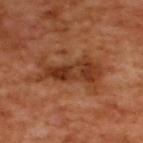Q: Was a biopsy performed?
A: catalogued during a skin exam; not biopsied
Q: Patient demographics?
A: male, aged 48 to 52
Q: What kind of image is this?
A: total-body-photography crop, ~15 mm field of view
Q: Where on the body is the lesion?
A: the upper back
Q: What is the lesion's diameter?
A: ~7.5 mm (longest diameter)
Q: What did automated image analysis measure?
A: a mean CIELAB color near L≈37 a*≈25 b*≈34, roughly 10 lightness units darker than nearby skin, and a normalized lesion–skin contrast near 8; an automated nevus-likeness rating near 50 out of 100 and a detector confidence of about 100 out of 100 that the crop contains a lesion